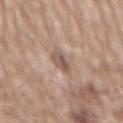The lesion was photographed on a routine skin check and not biopsied; there is no pathology result. The lesion-visualizer software estimated a lesion color around L≈54 a*≈17 b*≈25 in CIELAB, about 11 CIELAB-L* units darker than the surrounding skin, and a normalized border contrast of about 7.5. It also reported a nevus-likeness score of about 0/100 and a detector confidence of about 90 out of 100 that the crop contains a lesion. Cropped from a whole-body photographic skin survey; the tile spans about 15 mm. The lesion is on the abdomen. A male subject about 60 years old.